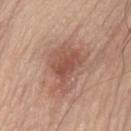An algorithmic analysis of the crop reported border irregularity of about 5.5 on a 0–10 scale and a within-lesion color-variation index near 4.5/10. Captured under white-light illumination. The lesion is on the chest. Cropped from a whole-body photographic skin survey; the tile spans about 15 mm. The lesion's longest dimension is about 5.5 mm. The patient is a male about 55 years old.